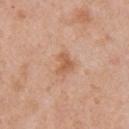illumination: white-light illumination | imaging modality: 15 mm crop, total-body photography | anatomic site: the right upper arm | TBP lesion metrics: a border-irregularity index near 5/10 and a within-lesion color-variation index near 1.5/10 | subject: female, in their 40s | diameter: ≈2.5 mm.